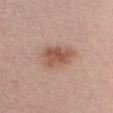Clinical impression:
Imaged during a routine full-body skin examination; the lesion was not biopsied and no histopathology is available.
Context:
The tile uses white-light illumination. The lesion-visualizer software estimated an eccentricity of roughly 0.75 and two-axis asymmetry of about 0.2. And it measured an average lesion color of about L≈55 a*≈22 b*≈28 (CIELAB) and a normalized border contrast of about 7.5. And it measured a border-irregularity rating of about 2.5/10 and a color-variation rating of about 4/10. The lesion is located on the chest. Cropped from a total-body skin-imaging series; the visible field is about 15 mm. A female subject aged around 25.